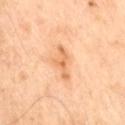Impression: Imaged during a routine full-body skin examination; the lesion was not biopsied and no histopathology is available. Acquisition and patient details: A region of skin cropped from a whole-body photographic capture, roughly 15 mm wide. The subject is a male aged around 70. Longest diameter approximately 4 mm. On the right thigh. The tile uses cross-polarized illumination.